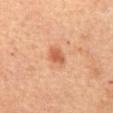Assessment: Recorded during total-body skin imaging; not selected for excision or biopsy. Clinical summary: A female subject approximately 60 years of age. Located on the chest. The lesion-visualizer software estimated internal color variation of about 1.5 on a 0–10 scale and peripheral color asymmetry of about 0.5. A 15 mm crop from a total-body photograph taken for skin-cancer surveillance. The tile uses cross-polarized illumination. Longest diameter approximately 2.5 mm.